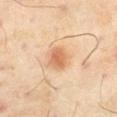- lighting · cross-polarized
- anatomic site · the right thigh
- automated lesion analysis · a border-irregularity index near 2/10
- acquisition · total-body-photography crop, ~15 mm field of view
- subject · male, in their mid- to late 50s